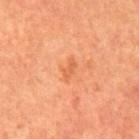Q: Is there a histopathology result?
A: total-body-photography surveillance lesion; no biopsy
Q: What kind of image is this?
A: 15 mm crop, total-body photography
Q: Patient demographics?
A: male, in their mid-60s
Q: Where on the body is the lesion?
A: the abdomen
Q: Illumination type?
A: cross-polarized illumination